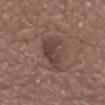The lesion was tiled from a total-body skin photograph and was not biopsied. A 15 mm close-up tile from a total-body photography series done for melanoma screening. A male patient, approximately 80 years of age. The recorded lesion diameter is about 5.5 mm. The lesion is on the chest. Imaged with white-light lighting. Automated image analysis of the tile measured a lesion color around L≈41 a*≈17 b*≈22 in CIELAB, about 8 CIELAB-L* units darker than the surrounding skin, and a normalized border contrast of about 7. The analysis additionally found radial color variation of about 1.5.